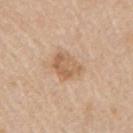Clinical impression:
The lesion was photographed on a routine skin check and not biopsied; there is no pathology result.
Clinical summary:
Approximately 4 mm at its widest. The lesion is on the left upper arm. A 15 mm close-up tile from a total-body photography series done for melanoma screening. The tile uses white-light illumination. The patient is a male aged approximately 60.